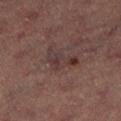The lesion is on the left lower leg.
The patient is a female aged 68–72.
A region of skin cropped from a whole-body photographic capture, roughly 15 mm wide.
Imaged with cross-polarized lighting.